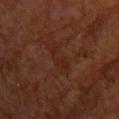This lesion was catalogued during total-body skin photography and was not selected for biopsy. A male subject roughly 65 years of age. Longest diameter approximately 3.5 mm. This is a cross-polarized tile. The lesion is located on the upper back. A 15 mm crop from a total-body photograph taken for skin-cancer surveillance.